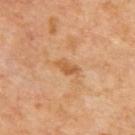| feature | finding |
|---|---|
| workup | imaged on a skin check; not biopsied |
| anatomic site | the upper back |
| subject | aged 63–67 |
| image source | 15 mm crop, total-body photography |